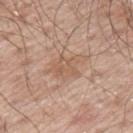Image and clinical context: Cropped from a total-body skin-imaging series; the visible field is about 15 mm. An algorithmic analysis of the crop reported an average lesion color of about L≈58 a*≈18 b*≈30 (CIELAB), about 7 CIELAB-L* units darker than the surrounding skin, and a normalized border contrast of about 5.5. Imaged with white-light lighting. Longest diameter approximately 5 mm. From the left upper arm. A male patient, in their mid-60s.